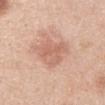The lesion was photographed on a routine skin check and not biopsied; there is no pathology result.